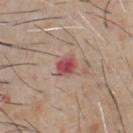  biopsy_status: not biopsied; imaged during a skin examination
  lighting: white-light
  patient:
    sex: male
    age_approx: 60
  image:
    source: total-body photography crop
    field_of_view_mm: 15
  lesion_size:
    long_diameter_mm_approx: 2.5
  site: chest